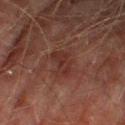Case summary:
• follow-up: no biopsy performed (imaged during a skin exam)
• location: the right thigh
• lighting: cross-polarized illumination
• patient: male, aged 73 to 77
• imaging modality: ~15 mm tile from a whole-body skin photo
• TBP lesion metrics: a shape-asymmetry score of about 0.35 (0 = symmetric); an average lesion color of about L≈25 a*≈19 b*≈20 (CIELAB), about 5 CIELAB-L* units darker than the surrounding skin, and a normalized border contrast of about 6; a classifier nevus-likeness of about 0/100 and a lesion-detection confidence of about 100/100
• lesion size: about 3 mm